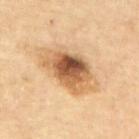A male patient, roughly 85 years of age or older.
Captured under cross-polarized illumination.
Cropped from a whole-body photographic skin survey; the tile spans about 15 mm.
Located on the mid back.
The recorded lesion diameter is about 8 mm.